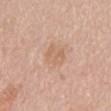Imaged during a routine full-body skin examination; the lesion was not biopsied and no histopathology is available. Located on the back. Cropped from a total-body skin-imaging series; the visible field is about 15 mm. A female patient, in their mid-60s. Captured under white-light illumination.